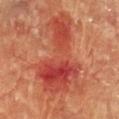Assessment:
Imaged during a routine full-body skin examination; the lesion was not biopsied and no histopathology is available.
Acquisition and patient details:
A female subject aged 78–82. A roughly 15 mm field-of-view crop from a total-body skin photograph. The lesion is on the chest. This is a cross-polarized tile. The recorded lesion diameter is about 10.5 mm. Automated tile analysis of the lesion measured a shape eccentricity near 0.9 and a symmetry-axis asymmetry near 0.6. It also reported a lesion color around L≈43 a*≈34 b*≈32 in CIELAB, roughly 10 lightness units darker than nearby skin, and a normalized border contrast of about 7.5. The software also gave a nevus-likeness score of about 0/100 and lesion-presence confidence of about 100/100.